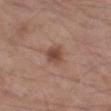Assessment:
The lesion was photographed on a routine skin check and not biopsied; there is no pathology result.
Background:
The tile uses white-light illumination. Longest diameter approximately 2.5 mm. The subject is a male approximately 55 years of age. The lesion-visualizer software estimated an average lesion color of about L≈47 a*≈21 b*≈27 (CIELAB), a lesion–skin lightness drop of about 10, and a normalized lesion–skin contrast near 7.5. The software also gave a color-variation rating of about 3.5/10. And it measured an automated nevus-likeness rating near 70 out of 100. A 15 mm close-up extracted from a 3D total-body photography capture. On the left thigh.